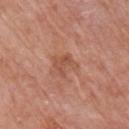lighting: white-light illumination
acquisition: total-body-photography crop, ~15 mm field of view
location: the right upper arm
size: about 2.5 mm
subject: male, approximately 80 years of age
automated lesion analysis: a border-irregularity rating of about 5.5/10 and a peripheral color-asymmetry measure near 1; a lesion-detection confidence of about 100/100